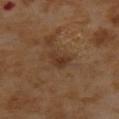notes = total-body-photography surveillance lesion; no biopsy | image source = ~15 mm tile from a whole-body skin photo | subject = female, approximately 55 years of age | body site = the upper back | diameter = about 3 mm | image-analysis metrics = internal color variation of about 2.5 on a 0–10 scale and peripheral color asymmetry of about 1.